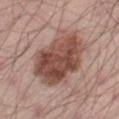{"biopsy_status": "not biopsied; imaged during a skin examination", "image": {"source": "total-body photography crop", "field_of_view_mm": 15}, "site": "right thigh", "patient": {"sex": "male", "age_approx": 45}, "automated_metrics": {"eccentricity": 0.75, "shape_asymmetry": 0.2, "cielab_L": 48, "cielab_a": 21, "cielab_b": 25, "vs_skin_darker_L": 14.0, "nevus_likeness_0_100": 20, "lesion_detection_confidence_0_100": 100}}